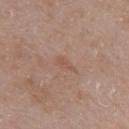The lesion was photographed on a routine skin check and not biopsied; there is no pathology result. A 15 mm close-up extracted from a 3D total-body photography capture. From the left upper arm. The subject is a female about 55 years old. The lesion-visualizer software estimated a mean CIELAB color near L≈53 a*≈18 b*≈29. It also reported a nevus-likeness score of about 0/100 and a lesion-detection confidence of about 100/100. Captured under white-light illumination. About 2.5 mm across.